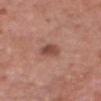notes — catalogued during a skin exam; not biopsied | patient — male, aged 58 to 62 | automated lesion analysis — an eccentricity of roughly 0.7 and a symmetry-axis asymmetry near 0.2; a mean CIELAB color near L≈48 a*≈24 b*≈26, a lesion–skin lightness drop of about 11, and a normalized border contrast of about 7.5; a border-irregularity index near 2/10 and radial color variation of about 1.5; a nevus-likeness score of about 70/100 and lesion-presence confidence of about 100/100 | body site — the head or neck | imaging modality — ~15 mm tile from a whole-body skin photo | diameter — about 3 mm.